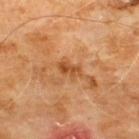Imaged during a routine full-body skin examination; the lesion was not biopsied and no histopathology is available.
Automated image analysis of the tile measured an area of roughly 3.5 mm², a shape eccentricity near 0.85, and two-axis asymmetry of about 0.35. And it measured a mean CIELAB color near L≈49 a*≈25 b*≈41, about 10 CIELAB-L* units darker than the surrounding skin, and a normalized lesion–skin contrast near 7.5. The analysis additionally found border irregularity of about 3.5 on a 0–10 scale, internal color variation of about 3.5 on a 0–10 scale, and a peripheral color-asymmetry measure near 1.5. And it measured an automated nevus-likeness rating near 0 out of 100 and a detector confidence of about 100 out of 100 that the crop contains a lesion.
A male subject, aged 58 to 62.
The tile uses cross-polarized illumination.
From the chest.
A lesion tile, about 15 mm wide, cut from a 3D total-body photograph.
About 3 mm across.